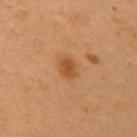Imaged during a routine full-body skin examination; the lesion was not biopsied and no histopathology is available.
The subject is a female about 60 years old.
A 15 mm crop from a total-body photograph taken for skin-cancer surveillance.
From the chest.